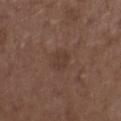Findings:
* biopsy status: no biopsy performed (imaged during a skin exam)
* lesion size: about 3 mm
* subject: female, aged around 35
* site: the upper back
* image source: total-body-photography crop, ~15 mm field of view
* automated metrics: an area of roughly 5 mm², an eccentricity of roughly 0.65, and two-axis asymmetry of about 0.15; a color-variation rating of about 1.5/10 and a peripheral color-asymmetry measure near 0.5
* tile lighting: white-light